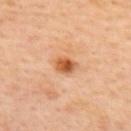Case summary:
• biopsy status — total-body-photography surveillance lesion; no biopsy
• size — about 2.5 mm
• body site — the upper back
• imaging modality — total-body-photography crop, ~15 mm field of view
• lighting — cross-polarized
• subject — female, about 40 years old